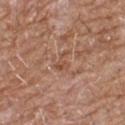Imaged during a routine full-body skin examination; the lesion was not biopsied and no histopathology is available.
A male patient, aged around 60.
On the chest.
This is a white-light tile.
About 3 mm across.
The total-body-photography lesion software estimated an average lesion color of about L≈50 a*≈21 b*≈31 (CIELAB), a lesion–skin lightness drop of about 7, and a normalized border contrast of about 5.5. It also reported a border-irregularity rating of about 6/10, a color-variation rating of about 1/10, and peripheral color asymmetry of about 0.5. The analysis additionally found an automated nevus-likeness rating near 0 out of 100 and a lesion-detection confidence of about 80/100.
A close-up tile cropped from a whole-body skin photograph, about 15 mm across.